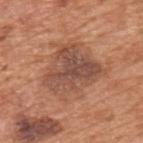Findings:
• follow-up: catalogued during a skin exam; not biopsied
• body site: the left upper arm
• imaging modality: ~15 mm crop, total-body skin-cancer survey
• automated lesion analysis: border irregularity of about 4 on a 0–10 scale and internal color variation of about 5 on a 0–10 scale
• tile lighting: white-light
• size: ~6.5 mm (longest diameter)
• subject: male, in their mid- to late 60s